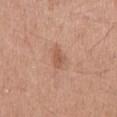No biopsy was performed on this lesion — it was imaged during a full skin examination and was not determined to be concerning. A male subject, aged 28 to 32. A 15 mm close-up extracted from a 3D total-body photography capture. On the mid back. The tile uses white-light illumination. Longest diameter approximately 2.5 mm.